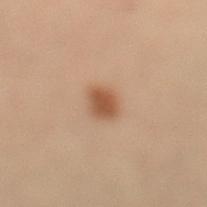imaging modality: ~15 mm crop, total-body skin-cancer survey; location: the left lower leg; subject: female, aged 38–42; lighting: cross-polarized illumination.